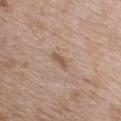| field | value |
|---|---|
| notes | catalogued during a skin exam; not biopsied |
| anatomic site | the chest |
| image | ~15 mm crop, total-body skin-cancer survey |
| lesion size | ~3 mm (longest diameter) |
| illumination | white-light illumination |
| patient | male, in their 50s |